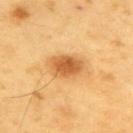Assessment:
This lesion was catalogued during total-body skin photography and was not selected for biopsy.
Image and clinical context:
The tile uses cross-polarized illumination. A 15 mm close-up extracted from a 3D total-body photography capture. The lesion is located on the right upper arm. Longest diameter approximately 4 mm. The total-body-photography lesion software estimated an outline eccentricity of about 0.8 (0 = round, 1 = elongated) and a shape-asymmetry score of about 0.2 (0 = symmetric). The analysis additionally found a normalized border contrast of about 8.5. And it measured a border-irregularity rating of about 2/10, a within-lesion color-variation index near 3.5/10, and peripheral color asymmetry of about 1. It also reported a classifier nevus-likeness of about 95/100 and lesion-presence confidence of about 100/100. A male patient roughly 60 years of age.